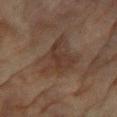* follow-up · catalogued during a skin exam; not biopsied
* subject · female, aged 78 to 82
* lighting · cross-polarized illumination
* site · the right forearm
* image source · total-body-photography crop, ~15 mm field of view
* size · ~6.5 mm (longest diameter)